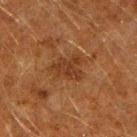Captured during whole-body skin photography for melanoma surveillance; the lesion was not biopsied.
On the right upper arm.
A 15 mm close-up tile from a total-body photography series done for melanoma screening.
This is a cross-polarized tile.
The subject is a male roughly 60 years of age.